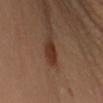Assessment: Recorded during total-body skin imaging; not selected for excision or biopsy. Context: Cropped from a total-body skin-imaging series; the visible field is about 15 mm. An algorithmic analysis of the crop reported an average lesion color of about L≈33 a*≈21 b*≈28 (CIELAB), a lesion–skin lightness drop of about 10, and a normalized border contrast of about 9.5. The patient is a female in their 30s. Located on the left upper arm. Captured under cross-polarized illumination.